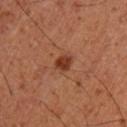Assessment:
Captured during whole-body skin photography for melanoma surveillance; the lesion was not biopsied.
Context:
Measured at roughly 2 mm in maximum diameter. The patient is a male aged 48 to 52. Imaged with cross-polarized lighting. On the arm. A 15 mm close-up extracted from a 3D total-body photography capture.